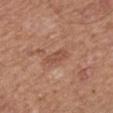Q: Was this lesion biopsied?
A: catalogued during a skin exam; not biopsied
Q: What is the imaging modality?
A: ~15 mm crop, total-body skin-cancer survey
Q: What lighting was used for the tile?
A: white-light illumination
Q: How large is the lesion?
A: about 3 mm
Q: Lesion location?
A: the back
Q: What are the patient's age and sex?
A: male, aged approximately 65
Q: Automated lesion metrics?
A: a lesion area of about 3.5 mm², an eccentricity of roughly 0.85, and a symmetry-axis asymmetry near 0.25; a nevus-likeness score of about 0/100 and lesion-presence confidence of about 100/100